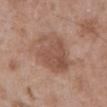Clinical impression: This lesion was catalogued during total-body skin photography and was not selected for biopsy. Background: A close-up tile cropped from a whole-body skin photograph, about 15 mm across. Located on the abdomen. Longest diameter approximately 5 mm. A male patient, aged 68 to 72.